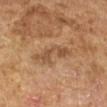The lesion was photographed on a routine skin check and not biopsied; there is no pathology result.
The tile uses cross-polarized illumination.
The lesion's longest dimension is about 4.5 mm.
A female subject, about 60 years old.
On the arm.
A roughly 15 mm field-of-view crop from a total-body skin photograph.